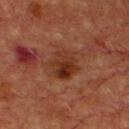- anatomic site: the chest
- tile lighting: cross-polarized
- patient: male, aged 63 to 67
- image: ~15 mm crop, total-body skin-cancer survey